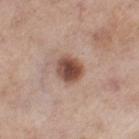| key | value |
|---|---|
| biopsy status | no biopsy performed (imaged during a skin exam) |
| image | 15 mm crop, total-body photography |
| patient | female, aged 53 to 57 |
| anatomic site | the left thigh |
| lesion size | about 3 mm |